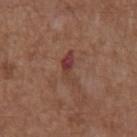The lesion was photographed on a routine skin check and not biopsied; there is no pathology result.
The tile uses white-light illumination.
A male subject, approximately 75 years of age.
Measured at roughly 4 mm in maximum diameter.
The lesion is on the front of the torso.
Cropped from a total-body skin-imaging series; the visible field is about 15 mm.